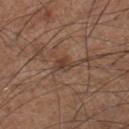<record>
<patient>
  <sex>male</sex>
  <age_approx>60</age_approx>
</patient>
<lesion_size>
  <long_diameter_mm_approx>2.5</long_diameter_mm_approx>
</lesion_size>
<image>
  <source>total-body photography crop</source>
  <field_of_view_mm>15</field_of_view_mm>
</image>
<automated_metrics>
  <area_mm2_approx>4.0</area_mm2_approx>
  <eccentricity>0.7</eccentricity>
  <shape_asymmetry>0.35</shape_asymmetry>
  <nevus_likeness_0_100>10</nevus_likeness_0_100>
  <lesion_detection_confidence_0_100>70</lesion_detection_confidence_0_100>
</automated_metrics>
<site>right lower leg</site>
</record>